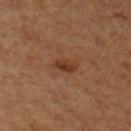Q: Where on the body is the lesion?
A: the chest
Q: Lesion size?
A: ≈3 mm
Q: What are the patient's age and sex?
A: male, aged 53 to 57
Q: What kind of image is this?
A: ~15 mm crop, total-body skin-cancer survey
Q: Illumination type?
A: cross-polarized illumination